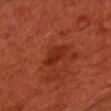Notes:
* workup · imaged on a skin check; not biopsied
* site · the chest
* lesion diameter · about 3.5 mm
* image · ~15 mm crop, total-body skin-cancer survey
* illumination · cross-polarized illumination
* patient · male, about 45 years old